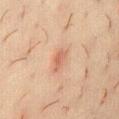<record>
<biopsy_status>not biopsied; imaged during a skin examination</biopsy_status>
<image>
  <source>total-body photography crop</source>
  <field_of_view_mm>15</field_of_view_mm>
</image>
<patient>
  <sex>female</sex>
  <age_approx>20</age_approx>
</patient>
<lesion_size>
  <long_diameter_mm_approx>3.0</long_diameter_mm_approx>
</lesion_size>
<site>abdomen</site>
<lighting>cross-polarized</lighting>
</record>